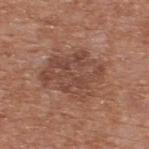Case summary:
• biopsy status · no biopsy performed (imaged during a skin exam)
• diameter · about 7.5 mm
• patient · male, aged around 65
• body site · the upper back
• image source · ~15 mm crop, total-body skin-cancer survey
• illumination · white-light